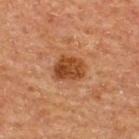| field | value |
|---|---|
| biopsy status | catalogued during a skin exam; not biopsied |